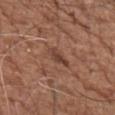Assessment:
The lesion was photographed on a routine skin check and not biopsied; there is no pathology result.
Clinical summary:
From the left upper arm. A close-up tile cropped from a whole-body skin photograph, about 15 mm across. The tile uses white-light illumination. A male patient in their mid- to late 70s. The lesion-visualizer software estimated a classifier nevus-likeness of about 0/100 and lesion-presence confidence of about 100/100. Approximately 3.5 mm at its widest.